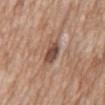Impression:
Recorded during total-body skin imaging; not selected for excision or biopsy.
Acquisition and patient details:
The lesion-visualizer software estimated a footprint of about 5.5 mm² and a shape-asymmetry score of about 0.2 (0 = symmetric). The analysis additionally found a mean CIELAB color near L≈48 a*≈19 b*≈26, roughly 13 lightness units darker than nearby skin, and a lesion-to-skin contrast of about 9.5 (normalized; higher = more distinct). The lesion is located on the front of the torso. A male patient, aged 58–62. Captured under white-light illumination. A roughly 15 mm field-of-view crop from a total-body skin photograph.